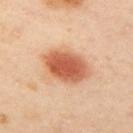Assessment: Part of a total-body skin-imaging series; this lesion was reviewed on a skin check and was not flagged for biopsy. Clinical summary: A 15 mm close-up tile from a total-body photography series done for melanoma screening. A female patient, aged around 40. This is a cross-polarized tile. Longest diameter approximately 6 mm. The lesion is located on the left arm. An algorithmic analysis of the crop reported an average lesion color of about L≈61 a*≈28 b*≈37 (CIELAB), about 16 CIELAB-L* units darker than the surrounding skin, and a normalized lesion–skin contrast near 10. And it measured an automated nevus-likeness rating near 100 out of 100 and a lesion-detection confidence of about 100/100.